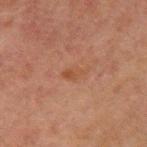Part of a total-body skin-imaging series; this lesion was reviewed on a skin check and was not flagged for biopsy. A male patient aged around 60. Located on the left upper arm. Automated image analysis of the tile measured a footprint of about 3 mm², an eccentricity of roughly 0.85, and a symmetry-axis asymmetry near 0.3. The analysis additionally found a mean CIELAB color near L≈37 a*≈19 b*≈27, about 5 CIELAB-L* units darker than the surrounding skin, and a normalized border contrast of about 5.5. It also reported a border-irregularity index near 3.5/10, internal color variation of about 1.5 on a 0–10 scale, and peripheral color asymmetry of about 0. A region of skin cropped from a whole-body photographic capture, roughly 15 mm wide.